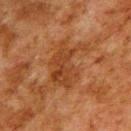notes = total-body-photography surveillance lesion; no biopsy | image-analysis metrics = an area of roughly 15 mm² and two-axis asymmetry of about 0.4; border irregularity of about 6.5 on a 0–10 scale, a color-variation rating of about 3.5/10, and peripheral color asymmetry of about 1 | lighting = cross-polarized illumination | body site = the upper back | patient = male, about 80 years old | image source = total-body-photography crop, ~15 mm field of view.